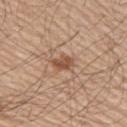{"biopsy_status": "not biopsied; imaged during a skin examination", "site": "right upper arm", "image": {"source": "total-body photography crop", "field_of_view_mm": 15}, "lighting": "white-light", "automated_metrics": {"area_mm2_approx": 4.0, "eccentricity": 0.75, "shape_asymmetry": 0.25, "nevus_likeness_0_100": 90, "lesion_detection_confidence_0_100": 100}, "lesion_size": {"long_diameter_mm_approx": 2.5}, "patient": {"sex": "male", "age_approx": 55}}